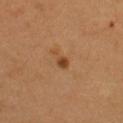This lesion was catalogued during total-body skin photography and was not selected for biopsy. The tile uses cross-polarized illumination. Cropped from a total-body skin-imaging series; the visible field is about 15 mm. The lesion's longest dimension is about 2.5 mm. The lesion is located on the chest. The patient is a female in their 40s. Automated tile analysis of the lesion measured a lesion color around L≈45 a*≈22 b*≈37 in CIELAB, about 9 CIELAB-L* units darker than the surrounding skin, and a normalized border contrast of about 7.5. It also reported a classifier nevus-likeness of about 80/100 and a lesion-detection confidence of about 100/100.